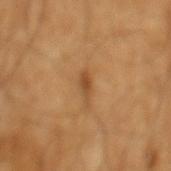Q: Was this lesion biopsied?
A: imaged on a skin check; not biopsied
Q: Lesion size?
A: about 3 mm
Q: Who is the patient?
A: male, approximately 65 years of age
Q: How was this image acquired?
A: 15 mm crop, total-body photography